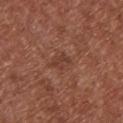Recorded during total-body skin imaging; not selected for excision or biopsy.
A female patient about 75 years old.
The tile uses white-light illumination.
Longest diameter approximately 2.5 mm.
Automated image analysis of the tile measured a lesion area of about 4 mm², a shape eccentricity near 0.7, and a shape-asymmetry score of about 0.3 (0 = symmetric).
A 15 mm crop from a total-body photograph taken for skin-cancer surveillance.
On the chest.